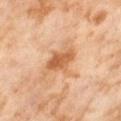biopsy status — imaged on a skin check; not biopsied
tile lighting — cross-polarized illumination
body site — the right thigh
image-analysis metrics — a lesion area of about 7 mm² and a shape eccentricity near 0.65; a mean CIELAB color near L≈61 a*≈25 b*≈41, a lesion–skin lightness drop of about 12, and a normalized border contrast of about 8; internal color variation of about 3.5 on a 0–10 scale and a peripheral color-asymmetry measure near 1; an automated nevus-likeness rating near 25 out of 100 and lesion-presence confidence of about 100/100
image source — ~15 mm tile from a whole-body skin photo
subject — female, aged 53–57
diameter — ~3.5 mm (longest diameter)